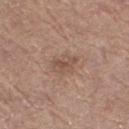- workup — imaged on a skin check; not biopsied
- image-analysis metrics — a detector confidence of about 100 out of 100 that the crop contains a lesion
- tile lighting — white-light
- acquisition — ~15 mm tile from a whole-body skin photo
- patient — male, in their mid- to late 60s
- body site — the right thigh
- size — ≈2.5 mm